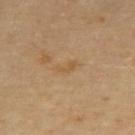Q: Was this lesion biopsied?
A: catalogued during a skin exam; not biopsied
Q: What did automated image analysis measure?
A: an area of roughly 2.5 mm² and two-axis asymmetry of about 0.45; a color-variation rating of about 0/10 and peripheral color asymmetry of about 0; an automated nevus-likeness rating near 0 out of 100
Q: Illumination type?
A: cross-polarized illumination
Q: What is the anatomic site?
A: the back
Q: What is the lesion's diameter?
A: ~2.5 mm (longest diameter)
Q: What are the patient's age and sex?
A: female, aged approximately 70
Q: What is the imaging modality?
A: total-body-photography crop, ~15 mm field of view